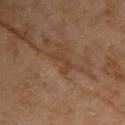Part of a total-body skin-imaging series; this lesion was reviewed on a skin check and was not flagged for biopsy. A female patient, in their 60s. The recorded lesion diameter is about 3 mm. From the upper back. Captured under cross-polarized illumination. A lesion tile, about 15 mm wide, cut from a 3D total-body photograph.